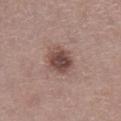Imaged during a routine full-body skin examination; the lesion was not biopsied and no histopathology is available. An algorithmic analysis of the crop reported a mean CIELAB color near L≈46 a*≈19 b*≈22 and a normalized border contrast of about 9.5. It also reported a border-irregularity rating of about 1.5/10, a color-variation rating of about 4.5/10, and radial color variation of about 1.5. Located on the leg. A female subject, aged 48–52. Imaged with white-light lighting. A 15 mm close-up extracted from a 3D total-body photography capture. About 3.5 mm across.